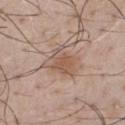Q: Automated lesion metrics?
A: a footprint of about 11 mm²; a lesion color around L≈56 a*≈17 b*≈27 in CIELAB and a lesion–skin lightness drop of about 8
Q: What lighting was used for the tile?
A: white-light illumination
Q: Patient demographics?
A: male, in their mid- to late 40s
Q: Where on the body is the lesion?
A: the chest
Q: How large is the lesion?
A: ~4 mm (longest diameter)
Q: What kind of image is this?
A: total-body-photography crop, ~15 mm field of view